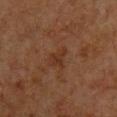The lesion was photographed on a routine skin check and not biopsied; there is no pathology result. A roughly 15 mm field-of-view crop from a total-body skin photograph. The lesion is located on the chest. The patient is a male aged 63–67.